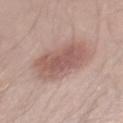notes — imaged on a skin check; not biopsied
site — the right thigh
image — ~15 mm tile from a whole-body skin photo
diameter — about 6 mm
patient — male, aged around 30